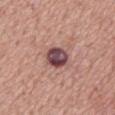Notes:
- workup · no biopsy performed (imaged during a skin exam)
- site · the back
- automated lesion analysis · internal color variation of about 8 on a 0–10 scale and peripheral color asymmetry of about 3
- lesion diameter · ≈3.5 mm
- acquisition · ~15 mm crop, total-body skin-cancer survey
- subject · male, aged approximately 70